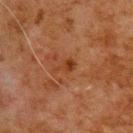<record>
<patient>
  <sex>male</sex>
  <age_approx>80</age_approx>
</patient>
<image>
  <source>total-body photography crop</source>
  <field_of_view_mm>15</field_of_view_mm>
</image>
<site>upper back</site>
</record>